Cropped from a total-body skin-imaging series; the visible field is about 15 mm · the lesion is located on the mid back · a female subject aged 63 to 67:
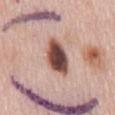histopathologic diagnosis = an atypical melanocytic neoplasm — a lesion of indeterminate malignant potential.